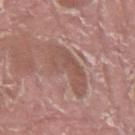notes = no biopsy performed (imaged during a skin exam)
automated metrics = a mean CIELAB color near L≈52 a*≈20 b*≈24 and roughly 8 lightness units darker than nearby skin
anatomic site = the left thigh
diameter = about 6.5 mm
acquisition = ~15 mm tile from a whole-body skin photo
subject = male, aged around 40